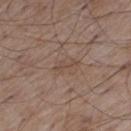{"biopsy_status": "not biopsied; imaged during a skin examination", "site": "left thigh", "lesion_size": {"long_diameter_mm_approx": 3.0}, "image": {"source": "total-body photography crop", "field_of_view_mm": 15}, "patient": {"sex": "male", "age_approx": 55}, "automated_metrics": {"vs_skin_darker_L": 6.0, "vs_skin_contrast_norm": 4.5, "border_irregularity_0_10": 6.0, "color_variation_0_10": 0.0, "peripheral_color_asymmetry": 0.0, "nevus_likeness_0_100": 0}, "lighting": "white-light"}